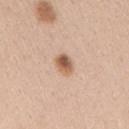Imaged during a routine full-body skin examination; the lesion was not biopsied and no histopathology is available.
A region of skin cropped from a whole-body photographic capture, roughly 15 mm wide.
An algorithmic analysis of the crop reported a lesion color around L≈59 a*≈20 b*≈31 in CIELAB and a normalized border contrast of about 9. It also reported an automated nevus-likeness rating near 100 out of 100 and a lesion-detection confidence of about 100/100.
Measured at roughly 2.5 mm in maximum diameter.
Located on the arm.
A female subject aged 38 to 42.